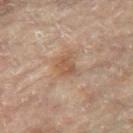The lesion was tiled from a total-body skin photograph and was not biopsied. The patient is a female roughly 80 years of age. An algorithmic analysis of the crop reported an outline eccentricity of about 0.65 (0 = round, 1 = elongated). The analysis additionally found radial color variation of about 0.5. And it measured an automated nevus-likeness rating near 35 out of 100. The tile uses cross-polarized illumination. Cropped from a total-body skin-imaging series; the visible field is about 15 mm. From the left leg. Approximately 3 mm at its widest.